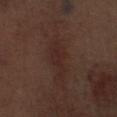Notes:
– notes — imaged on a skin check; not biopsied
– acquisition — ~15 mm crop, total-body skin-cancer survey
– automated lesion analysis — a lesion color around L≈25 a*≈18 b*≈20 in CIELAB and a lesion–skin lightness drop of about 5; a color-variation rating of about 1/10 and radial color variation of about 0.5
– body site — the right thigh
– lighting — white-light
– subject — male, approximately 70 years of age